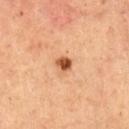Imaged during a routine full-body skin examination; the lesion was not biopsied and no histopathology is available. Cropped from a whole-body photographic skin survey; the tile spans about 15 mm. The subject is a male aged 63 to 67. The tile uses cross-polarized illumination. From the mid back. Measured at roughly 2 mm in maximum diameter.